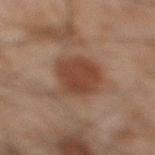Q: What kind of image is this?
A: ~15 mm crop, total-body skin-cancer survey
Q: Who is the patient?
A: male, in their mid-40s
Q: What lighting was used for the tile?
A: cross-polarized
Q: Lesion location?
A: the right lower leg